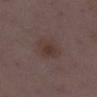The lesion was tiled from a total-body skin photograph and was not biopsied. A 15 mm close-up extracted from a 3D total-body photography capture. The lesion is located on the right lower leg. A female subject roughly 30 years of age.